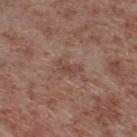{
  "biopsy_status": "not biopsied; imaged during a skin examination",
  "lighting": "white-light",
  "image": {
    "source": "total-body photography crop",
    "field_of_view_mm": 15
  },
  "lesion_size": {
    "long_diameter_mm_approx": 3.0
  },
  "patient": {
    "sex": "male",
    "age_approx": 55
  },
  "site": "left lower leg"
}